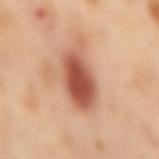A female subject, aged 53 to 57. Cropped from a whole-body photographic skin survey; the tile spans about 15 mm. The lesion is on the mid back. The lesion's longest dimension is about 6.5 mm. Captured under cross-polarized illumination.